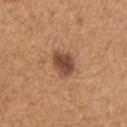Captured during whole-body skin photography for melanoma surveillance; the lesion was not biopsied. A male subject, approximately 65 years of age. Cropped from a total-body skin-imaging series; the visible field is about 15 mm. On the left upper arm.